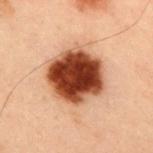A roughly 15 mm field-of-view crop from a total-body skin photograph. Automated image analysis of the tile measured a footprint of about 29 mm² and a shape eccentricity near 0.5. The analysis additionally found border irregularity of about 1.5 on a 0–10 scale and a peripheral color-asymmetry measure near 2.5. The software also gave a classifier nevus-likeness of about 100/100 and a lesion-detection confidence of about 100/100. The lesion is located on the right upper arm. The subject is a male aged 53–57. Measured at roughly 6.5 mm in maximum diameter. This is a cross-polarized tile.